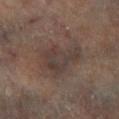follow-up = imaged on a skin check; not biopsied
anatomic site = the right lower leg
acquisition = total-body-photography crop, ~15 mm field of view
automated metrics = border irregularity of about 4.5 on a 0–10 scale, a color-variation rating of about 4.5/10, and a peripheral color-asymmetry measure near 1.5; an automated nevus-likeness rating near 0 out of 100 and a detector confidence of about 95 out of 100 that the crop contains a lesion
subject = male, about 65 years old
lesion size = ~5.5 mm (longest diameter)